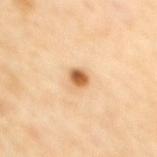- follow-up · no biopsy performed (imaged during a skin exam)
- patient · female, about 45 years old
- illumination · cross-polarized illumination
- image · total-body-photography crop, ~15 mm field of view
- diameter · about 2.5 mm
- location · the upper back
- automated metrics · a lesion area of about 4 mm², an outline eccentricity of about 0.55 (0 = round, 1 = elongated), and two-axis asymmetry of about 0.2; about 16 CIELAB-L* units darker than the surrounding skin and a normalized border contrast of about 10